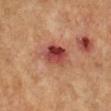{
  "biopsy_status": "not biopsied; imaged during a skin examination",
  "site": "left lower leg",
  "patient": {
    "sex": "female",
    "age_approx": 65
  },
  "lesion_size": {
    "long_diameter_mm_approx": 4.0
  },
  "image": {
    "source": "total-body photography crop",
    "field_of_view_mm": 15
  }
}